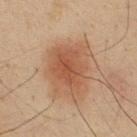Q: Was a biopsy performed?
A: no biopsy performed (imaged during a skin exam)
Q: What is the anatomic site?
A: the upper back
Q: What kind of image is this?
A: total-body-photography crop, ~15 mm field of view
Q: Patient demographics?
A: male, aged approximately 35
Q: Illumination type?
A: cross-polarized
Q: Lesion size?
A: about 5.5 mm
Q: Automated lesion metrics?
A: a border-irregularity index near 4.5/10 and internal color variation of about 3.5 on a 0–10 scale; lesion-presence confidence of about 100/100